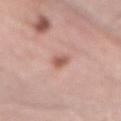follow-up = total-body-photography surveillance lesion; no biopsy
image source = ~15 mm tile from a whole-body skin photo
size = ~2.5 mm (longest diameter)
illumination = white-light illumination
image-analysis metrics = a color-variation rating of about 3.5/10 and a peripheral color-asymmetry measure near 1
body site = the mid back
subject = male, aged 63 to 67